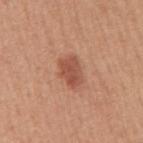| field | value |
|---|---|
| notes | imaged on a skin check; not biopsied |
| image | ~15 mm tile from a whole-body skin photo |
| location | the right upper arm |
| patient | male, aged 48 to 52 |